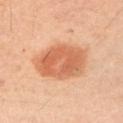workup — catalogued during a skin exam; not biopsied
size — ~6.5 mm (longest diameter)
TBP lesion metrics — a lesion area of about 22 mm², an outline eccentricity of about 0.75 (0 = round, 1 = elongated), and two-axis asymmetry of about 0.15; a mean CIELAB color near L≈61 a*≈26 b*≈37, about 12 CIELAB-L* units darker than the surrounding skin, and a normalized border contrast of about 8
location — the left upper arm
patient — male, approximately 40 years of age
lighting — cross-polarized
image source — ~15 mm tile from a whole-body skin photo